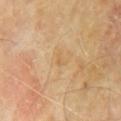<record>
  <patient>
    <sex>male</sex>
    <age_approx>60</age_approx>
  </patient>
  <image>
    <source>total-body photography crop</source>
    <field_of_view_mm>15</field_of_view_mm>
  </image>
  <site>chest</site>
  <diagnosis>
    <histopathology>seborrheic keratosis</histopathology>
    <malignancy>benign</malignancy>
    <taxonomic_path>Benign, Benign epidermal proliferations, Seborrheic keratosis</taxonomic_path>
  </diagnosis>
</record>